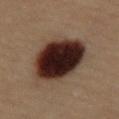notes: imaged on a skin check; not biopsied | patient: female, about 20 years old | image: 15 mm crop, total-body photography | anatomic site: the abdomen.